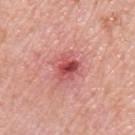Captured during whole-body skin photography for melanoma surveillance; the lesion was not biopsied.
The lesion is on the arm.
The recorded lesion diameter is about 3.5 mm.
A male subject in their 80s.
A 15 mm close-up extracted from a 3D total-body photography capture.
Captured under white-light illumination.